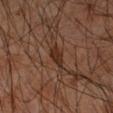Clinical impression: Captured during whole-body skin photography for melanoma surveillance; the lesion was not biopsied. Acquisition and patient details: Automated tile analysis of the lesion measured a footprint of about 4 mm², an outline eccentricity of about 0.85 (0 = round, 1 = elongated), and a shape-asymmetry score of about 0.25 (0 = symmetric). It also reported a mean CIELAB color near L≈25 a*≈17 b*≈23, about 8 CIELAB-L* units darker than the surrounding skin, and a normalized border contrast of about 9. The software also gave a color-variation rating of about 1.5/10 and a peripheral color-asymmetry measure near 0.5. And it measured an automated nevus-likeness rating near 5 out of 100 and a detector confidence of about 85 out of 100 that the crop contains a lesion. From the right forearm. Captured under cross-polarized illumination. A male patient, aged 63 to 67. A lesion tile, about 15 mm wide, cut from a 3D total-body photograph.